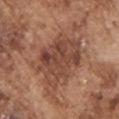Assessment: Captured during whole-body skin photography for melanoma surveillance; the lesion was not biopsied. Clinical summary: A close-up tile cropped from a whole-body skin photograph, about 15 mm across. The lesion is located on the chest. The lesion-visualizer software estimated a lesion area of about 26 mm², an eccentricity of roughly 0.65, and a symmetry-axis asymmetry near 0.25. And it measured a lesion color around L≈45 a*≈22 b*≈28 in CIELAB, about 10 CIELAB-L* units darker than the surrounding skin, and a normalized lesion–skin contrast near 7.5. The analysis additionally found a classifier nevus-likeness of about 5/100 and a detector confidence of about 100 out of 100 that the crop contains a lesion. A male patient in their mid- to late 70s. The lesion's longest dimension is about 6.5 mm.